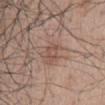Q: Is there a histopathology result?
A: catalogued during a skin exam; not biopsied
Q: What is the imaging modality?
A: ~15 mm crop, total-body skin-cancer survey
Q: Where on the body is the lesion?
A: the mid back
Q: What are the patient's age and sex?
A: male, aged 68–72